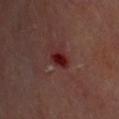Imaged during a routine full-body skin examination; the lesion was not biopsied and no histopathology is available.